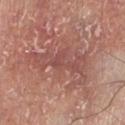follow-up — total-body-photography surveillance lesion; no biopsy | anatomic site — the leg | diameter — ~8.5 mm (longest diameter) | imaging modality — 15 mm crop, total-body photography | tile lighting — white-light | TBP lesion metrics — a lesion area of about 20 mm² and an outline eccentricity of about 0.9 (0 = round, 1 = elongated); a lesion color around L≈51 a*≈24 b*≈24 in CIELAB and about 8 CIELAB-L* units darker than the surrounding skin | subject — male, roughly 55 years of age.